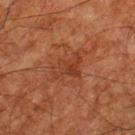Recorded during total-body skin imaging; not selected for excision or biopsy.
Automated image analysis of the tile measured a mean CIELAB color near L≈30 a*≈22 b*≈27. And it measured a border-irregularity index near 6/10 and internal color variation of about 4 on a 0–10 scale.
Located on the left thigh.
The subject is a male about 80 years old.
The lesion's longest dimension is about 3.5 mm.
The tile uses cross-polarized illumination.
A region of skin cropped from a whole-body photographic capture, roughly 15 mm wide.